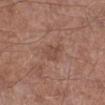Captured during whole-body skin photography for melanoma surveillance; the lesion was not biopsied.
Cropped from a whole-body photographic skin survey; the tile spans about 15 mm.
Approximately 2.5 mm at its widest.
Captured under white-light illumination.
The subject is a male approximately 65 years of age.
On the right lower leg.
An algorithmic analysis of the crop reported a nevus-likeness score of about 0/100 and lesion-presence confidence of about 100/100.